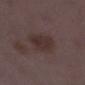Captured during whole-body skin photography for melanoma surveillance; the lesion was not biopsied.
On the left lower leg.
The lesion-visualizer software estimated a mean CIELAB color near L≈30 a*≈15 b*≈16, a lesion–skin lightness drop of about 7, and a normalized border contrast of about 7. It also reported a border-irregularity rating of about 2.5/10 and a within-lesion color-variation index near 2.5/10.
Measured at roughly 4.5 mm in maximum diameter.
A 15 mm close-up extracted from a 3D total-body photography capture.
The tile uses white-light illumination.
A female patient in their 30s.